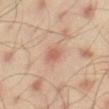Recorded during total-body skin imaging; not selected for excision or biopsy. A male subject, approximately 45 years of age. The total-body-photography lesion software estimated an area of roughly 8 mm², an eccentricity of roughly 0.75, and a symmetry-axis asymmetry near 0.15. The analysis additionally found an average lesion color of about L≈62 a*≈20 b*≈28 (CIELAB), a lesion–skin lightness drop of about 7, and a normalized lesion–skin contrast near 5. The software also gave border irregularity of about 1.5 on a 0–10 scale, internal color variation of about 4.5 on a 0–10 scale, and a peripheral color-asymmetry measure near 1. The recorded lesion diameter is about 4 mm. A roughly 15 mm field-of-view crop from a total-body skin photograph. The tile uses cross-polarized illumination. On the right thigh.